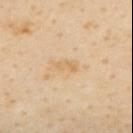Q: Is there a histopathology result?
A: catalogued during a skin exam; not biopsied
Q: What did automated image analysis measure?
A: a lesion area of about 3.5 mm² and a symmetry-axis asymmetry near 0.3; a lesion color around L≈70 a*≈16 b*≈41 in CIELAB and about 6 CIELAB-L* units darker than the surrounding skin; a border-irregularity index near 3/10, a color-variation rating of about 2/10, and peripheral color asymmetry of about 0.5
Q: How was this image acquired?
A: ~15 mm crop, total-body skin-cancer survey
Q: What are the patient's age and sex?
A: male, in their mid-50s
Q: How was the tile lit?
A: cross-polarized illumination
Q: Where on the body is the lesion?
A: the mid back